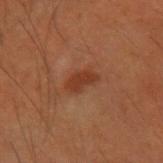biopsy_status: not biopsied; imaged during a skin examination
patient:
  sex: male
  age_approx: 50
lesion_size:
  long_diameter_mm_approx: 3.0
lighting: cross-polarized
site: right forearm
image:
  source: total-body photography crop
  field_of_view_mm: 15